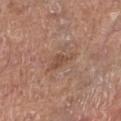Case summary:
* tile lighting: white-light illumination
* subject: male, aged 68–72
* anatomic site: the left lower leg
* image: ~15 mm tile from a whole-body skin photo
* lesion size: ~3.5 mm (longest diameter)